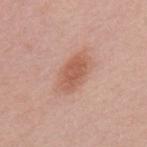Q: Is there a histopathology result?
A: imaged on a skin check; not biopsied
Q: What kind of image is this?
A: 15 mm crop, total-body photography
Q: What is the anatomic site?
A: the upper back
Q: Who is the patient?
A: female, roughly 60 years of age